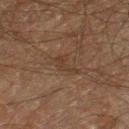Recorded during total-body skin imaging; not selected for excision or biopsy. From the leg. Cropped from a whole-body photographic skin survey; the tile spans about 15 mm. The lesion-visualizer software estimated an area of roughly 3 mm², an eccentricity of roughly 0.9, and two-axis asymmetry of about 0.65. The software also gave an average lesion color of about L≈27 a*≈13 b*≈22 (CIELAB), a lesion–skin lightness drop of about 4, and a normalized lesion–skin contrast near 5. This is a cross-polarized tile. Longest diameter approximately 3 mm. The patient is a male aged around 60.